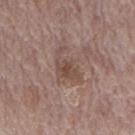A male subject, aged around 70.
Longest diameter approximately 4.5 mm.
The lesion-visualizer software estimated a mean CIELAB color near L≈49 a*≈16 b*≈23 and roughly 7 lightness units darker than nearby skin.
On the mid back.
Cropped from a total-body skin-imaging series; the visible field is about 15 mm.